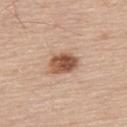  biopsy_status: not biopsied; imaged during a skin examination
  lighting: white-light
  lesion_size:
    long_diameter_mm_approx: 3.5
  site: upper back
  patient:
    sex: male
    age_approx: 80
  image:
    source: total-body photography crop
    field_of_view_mm: 15